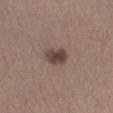Imaged during a routine full-body skin examination; the lesion was not biopsied and no histopathology is available.
Located on the left lower leg.
A close-up tile cropped from a whole-body skin photograph, about 15 mm across.
The subject is a female aged 38–42.